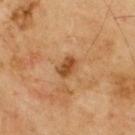workup: catalogued during a skin exam; not biopsied | diameter: about 2.5 mm | location: the upper back | illumination: cross-polarized | subject: male, about 70 years old | image: 15 mm crop, total-body photography.